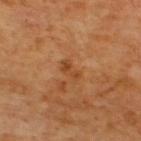biopsy_status: not biopsied; imaged during a skin examination